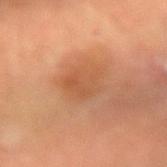notes: total-body-photography surveillance lesion; no biopsy
subject: male
image: 15 mm crop, total-body photography
location: the right forearm
tile lighting: cross-polarized